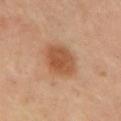Imaged during a routine full-body skin examination; the lesion was not biopsied and no histopathology is available.
The lesion is on the upper back.
This is a cross-polarized tile.
An algorithmic analysis of the crop reported an area of roughly 14 mm², a shape eccentricity near 0.7, and a symmetry-axis asymmetry near 0.15. And it measured an average lesion color of about L≈51 a*≈22 b*≈34 (CIELAB), a lesion–skin lightness drop of about 10, and a normalized lesion–skin contrast near 8. It also reported border irregularity of about 1.5 on a 0–10 scale and a peripheral color-asymmetry measure near 1.
A 15 mm close-up tile from a total-body photography series done for melanoma screening.
About 5 mm across.
A female subject, aged approximately 50.